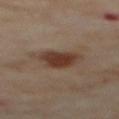biopsy status = no biopsy performed (imaged during a skin exam)
imaging modality = ~15 mm tile from a whole-body skin photo
illumination = cross-polarized
subject = female, aged 58 to 62
anatomic site = the upper back